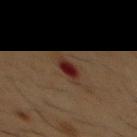The lesion was tiled from a total-body skin photograph and was not biopsied. The tile uses cross-polarized illumination. A male patient, aged 53 to 57. From the chest. Automated image analysis of the tile measured a border-irregularity rating of about 3.5/10, a within-lesion color-variation index near 6/10, and radial color variation of about 2. It also reported a nevus-likeness score of about 0/100 and a lesion-detection confidence of about 100/100. The recorded lesion diameter is about 3.5 mm. A region of skin cropped from a whole-body photographic capture, roughly 15 mm wide.